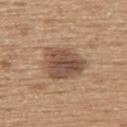Q: Was a biopsy performed?
A: no biopsy performed (imaged during a skin exam)
Q: What lighting was used for the tile?
A: white-light illumination
Q: What is the anatomic site?
A: the upper back
Q: How was this image acquired?
A: 15 mm crop, total-body photography
Q: Who is the patient?
A: male, in their mid-60s
Q: How large is the lesion?
A: about 5.5 mm